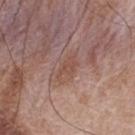workup: total-body-photography surveillance lesion; no biopsy | TBP lesion metrics: a lesion area of about 3.5 mm² and two-axis asymmetry of about 0.4; a lesion color around L≈49 a*≈20 b*≈26 in CIELAB, about 6 CIELAB-L* units darker than the surrounding skin, and a lesion-to-skin contrast of about 5.5 (normalized; higher = more distinct); a classifier nevus-likeness of about 0/100 and lesion-presence confidence of about 100/100 | lesion diameter: ~3 mm (longest diameter) | image source: ~15 mm tile from a whole-body skin photo | subject: male, aged approximately 65 | location: the back | illumination: white-light.